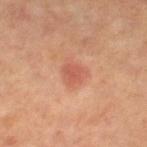Recorded during total-body skin imaging; not selected for excision or biopsy. Measured at roughly 3 mm in maximum diameter. On the left leg. Cropped from a whole-body photographic skin survey; the tile spans about 15 mm. Imaged with cross-polarized lighting. A female subject, roughly 65 years of age. Automated tile analysis of the lesion measured a footprint of about 7 mm², an outline eccentricity of about 0.55 (0 = round, 1 = elongated), and two-axis asymmetry of about 0.25. And it measured a border-irregularity rating of about 2.5/10, a within-lesion color-variation index near 2.5/10, and radial color variation of about 0.5.